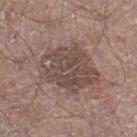Assessment: Recorded during total-body skin imaging; not selected for excision or biopsy. Clinical summary: Approximately 7.5 mm at its widest. A region of skin cropped from a whole-body photographic capture, roughly 15 mm wide. Imaged with white-light lighting. A male patient in their mid- to late 60s. On the right lower leg.